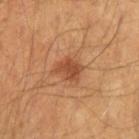Recorded during total-body skin imaging; not selected for excision or biopsy.
The subject is a male roughly 65 years of age.
Captured under cross-polarized illumination.
Automated tile analysis of the lesion measured about 10 CIELAB-L* units darker than the surrounding skin and a lesion-to-skin contrast of about 7.5 (normalized; higher = more distinct).
This image is a 15 mm lesion crop taken from a total-body photograph.
Located on the left forearm.
Measured at roughly 3 mm in maximum diameter.